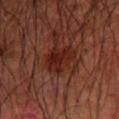Q: Was this lesion biopsied?
A: imaged on a skin check; not biopsied
Q: What are the patient's age and sex?
A: male, in their mid-60s
Q: What kind of image is this?
A: ~15 mm tile from a whole-body skin photo
Q: What is the lesion's diameter?
A: ~4 mm (longest diameter)
Q: What lighting was used for the tile?
A: cross-polarized
Q: Lesion location?
A: the left forearm